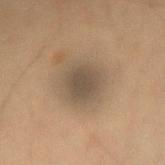notes: no biopsy performed (imaged during a skin exam)
imaging modality: total-body-photography crop, ~15 mm field of view
subject: male, aged 33–37
tile lighting: cross-polarized
site: the right forearm